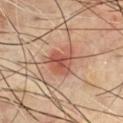| key | value |
|---|---|
| follow-up | catalogued during a skin exam; not biopsied |
| subject | male, in their 70s |
| site | the chest |
| image | total-body-photography crop, ~15 mm field of view |
| illumination | cross-polarized illumination |
| lesion size | ~3.5 mm (longest diameter) |